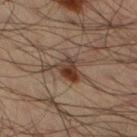  biopsy_status: not biopsied; imaged during a skin examination
  site: left lower leg
  patient:
    sex: male
    age_approx: 35
  image:
    source: total-body photography crop
    field_of_view_mm: 15
  automated_metrics:
    area_mm2_approx: 8.5
    eccentricity: 0.7
    shape_asymmetry: 0.6
    cielab_L: 35
    cielab_a: 15
    cielab_b: 24
    vs_skin_contrast_norm: 9.5
    border_irregularity_0_10: 7.0
    color_variation_0_10: 8.0
    peripheral_color_asymmetry: 2.5
  lesion_size:
    long_diameter_mm_approx: 4.5
  lighting: cross-polarized